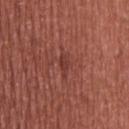Assessment: Recorded during total-body skin imaging; not selected for excision or biopsy. Context: The patient is a male approximately 45 years of age. A 15 mm close-up tile from a total-body photography series done for melanoma screening. On the chest. About 3 mm across. Captured under white-light illumination.